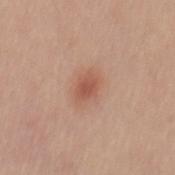Captured during whole-body skin photography for melanoma surveillance; the lesion was not biopsied.
About 3 mm across.
Cropped from a whole-body photographic skin survey; the tile spans about 15 mm.
A male subject, aged 28 to 32.
The tile uses white-light illumination.
An algorithmic analysis of the crop reported a footprint of about 5 mm², a shape eccentricity near 0.75, and two-axis asymmetry of about 0.35. The analysis additionally found a lesion color around L≈55 a*≈24 b*≈30 in CIELAB, a lesion–skin lightness drop of about 9, and a normalized lesion–skin contrast near 6.5. And it measured a border-irregularity index near 3/10, a color-variation rating of about 2.5/10, and peripheral color asymmetry of about 0.5.
From the mid back.